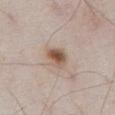A male subject aged 78–82. This is a white-light tile. The lesion-visualizer software estimated two-axis asymmetry of about 0.25. The analysis additionally found an automated nevus-likeness rating near 95 out of 100 and lesion-presence confidence of about 100/100. Cropped from a whole-body photographic skin survey; the tile spans about 15 mm. The lesion is on the abdomen. Measured at roughly 3 mm in maximum diameter.